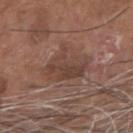| field | value |
|---|---|
| notes | total-body-photography surveillance lesion; no biopsy |
| diameter | ~5.5 mm (longest diameter) |
| patient | male, in their 70s |
| lighting | white-light |
| location | the head or neck |
| image | ~15 mm crop, total-body skin-cancer survey |
| automated lesion analysis | a lesion area of about 14 mm², an eccentricity of roughly 0.8, and a symmetry-axis asymmetry near 0.35 |